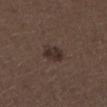biopsy status: total-body-photography surveillance lesion; no biopsy | image source: ~15 mm crop, total-body skin-cancer survey | automated lesion analysis: a footprint of about 5.5 mm², a shape eccentricity near 0.6, and two-axis asymmetry of about 0.2; a lesion color around L≈29 a*≈13 b*≈18 in CIELAB and a lesion-to-skin contrast of about 9 (normalized; higher = more distinct) | tile lighting: white-light illumination | subject: male, approximately 50 years of age | lesion size: about 3 mm | site: the leg.